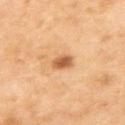A lesion tile, about 15 mm wide, cut from a 3D total-body photograph. The recorded lesion diameter is about 2.5 mm. The lesion is located on the upper back. The patient is a male in their mid-50s. The tile uses cross-polarized illumination.